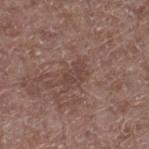{"site": "leg", "image": {"source": "total-body photography crop", "field_of_view_mm": 15}, "patient": {"sex": "male", "age_approx": 70}, "lesion_size": {"long_diameter_mm_approx": 3.0}}